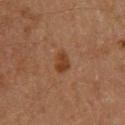The lesion was photographed on a routine skin check and not biopsied; there is no pathology result. Cropped from a total-body skin-imaging series; the visible field is about 15 mm. Longest diameter approximately 2.5 mm. A male subject, about 60 years old. The lesion-visualizer software estimated a lesion–skin lightness drop of about 8 and a normalized lesion–skin contrast near 8. The software also gave a detector confidence of about 100 out of 100 that the crop contains a lesion. Captured under cross-polarized illumination.